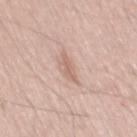<record>
  <biopsy_status>not biopsied; imaged during a skin examination</biopsy_status>
  <site>mid back</site>
  <image>
    <source>total-body photography crop</source>
    <field_of_view_mm>15</field_of_view_mm>
  </image>
  <patient>
    <sex>male</sex>
    <age_approx>40</age_approx>
  </patient>
  <lighting>white-light</lighting>
  <automated_metrics>
    <area_mm2_approx>3.0</area_mm2_approx>
    <eccentricity>0.95</eccentricity>
    <shape_asymmetry>0.3</shape_asymmetry>
    <cielab_L>63</cielab_L>
    <cielab_a>19</cielab_a>
    <cielab_b>26</cielab_b>
    <vs_skin_contrast_norm>6.0</vs_skin_contrast_norm>
    <border_irregularity_0_10>4.5</border_irregularity_0_10>
    <color_variation_0_10>0.0</color_variation_0_10>
    <peripheral_color_asymmetry>0.0</peripheral_color_asymmetry>
    <nevus_likeness_0_100>0</nevus_likeness_0_100>
    <lesion_detection_confidence_0_100>85</lesion_detection_confidence_0_100>
  </automated_metrics>
</record>